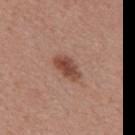Assessment: No biopsy was performed on this lesion — it was imaged during a full skin examination and was not determined to be concerning. Clinical summary: The lesion is located on the mid back. A male subject, aged approximately 55. Cropped from a total-body skin-imaging series; the visible field is about 15 mm. The tile uses white-light illumination.